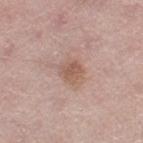Clinical impression: Imaged during a routine full-body skin examination; the lesion was not biopsied and no histopathology is available. Background: A lesion tile, about 15 mm wide, cut from a 3D total-body photograph. Imaged with white-light lighting. From the left thigh. An algorithmic analysis of the crop reported a shape eccentricity near 0.3 and two-axis asymmetry of about 0.25. It also reported a lesion–skin lightness drop of about 9 and a lesion-to-skin contrast of about 6.5 (normalized; higher = more distinct). The subject is a female roughly 60 years of age.